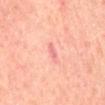- workup · catalogued during a skin exam; not biopsied
- image source · ~15 mm crop, total-body skin-cancer survey
- tile lighting · cross-polarized
- size · about 3 mm
- subject · male, about 70 years old
- location · the mid back